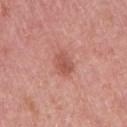Captured during whole-body skin photography for melanoma surveillance; the lesion was not biopsied.
A lesion tile, about 15 mm wide, cut from a 3D total-body photograph.
The lesion is located on the right upper arm.
A female patient, aged 48–52.